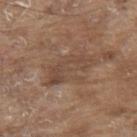Q: What is the imaging modality?
A: ~15 mm crop, total-body skin-cancer survey
Q: What is the lesion's diameter?
A: ≈6 mm
Q: Patient demographics?
A: male, aged 78 to 82
Q: Where on the body is the lesion?
A: the upper back